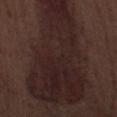<case>
<patient>
  <sex>male</sex>
  <age_approx>70</age_approx>
</patient>
<image>
  <source>total-body photography crop</source>
  <field_of_view_mm>15</field_of_view_mm>
</image>
<site>right lower leg</site>
</case>